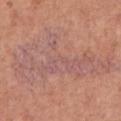| feature | finding |
|---|---|
| workup | imaged on a skin check; not biopsied |
| anatomic site | the chest |
| diameter | about 14 mm |
| image source | 15 mm crop, total-body photography |
| patient | male, aged 63–67 |
| automated metrics | a nevus-likeness score of about 0/100 and a lesion-detection confidence of about 55/100 |